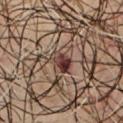Clinical impression:
Recorded during total-body skin imaging; not selected for excision or biopsy.
Acquisition and patient details:
The lesion is on the chest. A male subject aged approximately 65. The total-body-photography lesion software estimated a lesion area of about 5 mm², an outline eccentricity of about 0.75 (0 = round, 1 = elongated), and two-axis asymmetry of about 0.25. The analysis additionally found border irregularity of about 2.5 on a 0–10 scale. And it measured a lesion-detection confidence of about 100/100. Cropped from a total-body skin-imaging series; the visible field is about 15 mm. Longest diameter approximately 3 mm.